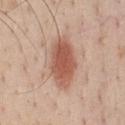Case summary:
– biopsy status — no biopsy performed (imaged during a skin exam)
– imaging modality — 15 mm crop, total-body photography
– anatomic site — the chest
– image-analysis metrics — a footprint of about 17 mm², a shape eccentricity near 0.85, and a symmetry-axis asymmetry near 0.15; a lesion color around L≈56 a*≈24 b*≈29 in CIELAB, roughly 13 lightness units darker than nearby skin, and a lesion-to-skin contrast of about 9 (normalized; higher = more distinct); a nevus-likeness score of about 100/100 and lesion-presence confidence of about 100/100
– patient — male, roughly 40 years of age
– lighting — white-light